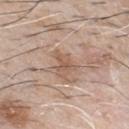Case summary:
- follow-up — catalogued during a skin exam; not biopsied
- lesion size — ~3.5 mm (longest diameter)
- location — the chest
- subject — male, in their mid-60s
- imaging modality — ~15 mm crop, total-body skin-cancer survey